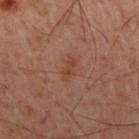  biopsy_status: not biopsied; imaged during a skin examination
  image:
    source: total-body photography crop
    field_of_view_mm: 15
  site: mid back
  patient:
    sex: male
    age_approx: 60